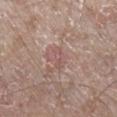workup = no biopsy performed (imaged during a skin exam) | acquisition = ~15 mm crop, total-body skin-cancer survey | image-analysis metrics = an eccentricity of roughly 0.9; a border-irregularity index near 3.5/10 and peripheral color asymmetry of about 0 | anatomic site = the leg | patient = male, approximately 80 years of age.